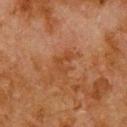Q: Was a biopsy performed?
A: catalogued during a skin exam; not biopsied
Q: Patient demographics?
A: male, aged around 80
Q: How was the tile lit?
A: cross-polarized illumination
Q: How was this image acquired?
A: ~15 mm crop, total-body skin-cancer survey
Q: Where on the body is the lesion?
A: the upper back
Q: What is the lesion's diameter?
A: ~3 mm (longest diameter)